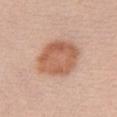{"biopsy_status": "not biopsied; imaged during a skin examination", "image": {"source": "total-body photography crop", "field_of_view_mm": 15}, "site": "left upper arm", "lighting": "white-light", "patient": {"sex": "female", "age_approx": 25}, "automated_metrics": {"cielab_L": 59, "cielab_a": 23, "cielab_b": 32, "vs_skin_darker_L": 12.0, "vs_skin_contrast_norm": 8.0, "border_irregularity_0_10": 1.5, "nevus_likeness_0_100": 90}}